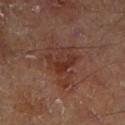{
  "biopsy_status": "not biopsied; imaged during a skin examination",
  "image": {
    "source": "total-body photography crop",
    "field_of_view_mm": 15
  },
  "patient": {
    "sex": "male",
    "age_approx": 70
  },
  "site": "leg"
}